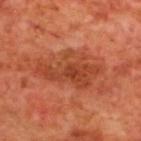Q: Is there a histopathology result?
A: catalogued during a skin exam; not biopsied
Q: How large is the lesion?
A: about 7 mm
Q: What is the anatomic site?
A: the back
Q: What are the patient's age and sex?
A: male, about 70 years old
Q: What kind of image is this?
A: ~15 mm crop, total-body skin-cancer survey